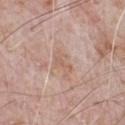Impression:
This lesion was catalogued during total-body skin photography and was not selected for biopsy.
Background:
The lesion is on the chest. A male patient aged 68–72. Cropped from a whole-body photographic skin survey; the tile spans about 15 mm. Automated image analysis of the tile measured border irregularity of about 4.5 on a 0–10 scale, a color-variation rating of about 1.5/10, and a peripheral color-asymmetry measure near 0.5. The lesion's longest dimension is about 3 mm. The tile uses white-light illumination.